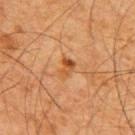Notes:
- notes: no biopsy performed (imaged during a skin exam)
- imaging modality: total-body-photography crop, ~15 mm field of view
- subject: male, roughly 65 years of age
- TBP lesion metrics: a footprint of about 3.5 mm², a shape eccentricity near 0.75, and a symmetry-axis asymmetry near 0.35; a mean CIELAB color near L≈43 a*≈22 b*≈36, a lesion–skin lightness drop of about 8, and a normalized lesion–skin contrast near 7.5; a peripheral color-asymmetry measure near 2; a lesion-detection confidence of about 100/100
- size: ≈2.5 mm
- anatomic site: the upper back